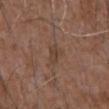Notes:
– subject — male, aged approximately 75
– anatomic site — the abdomen
– automated lesion analysis — lesion-presence confidence of about 100/100
– lesion diameter — about 3 mm
– illumination — white-light
– image — 15 mm crop, total-body photography